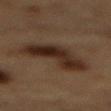Q: Was a biopsy performed?
A: imaged on a skin check; not biopsied
Q: Automated lesion metrics?
A: a footprint of about 20 mm², a shape eccentricity near 0.9, and a shape-asymmetry score of about 0.4 (0 = symmetric); an average lesion color of about L≈23 a*≈14 b*≈21 (CIELAB) and a normalized lesion–skin contrast near 11; a border-irregularity index near 4.5/10, a color-variation rating of about 5/10, and a peripheral color-asymmetry measure near 1.5; an automated nevus-likeness rating near 90 out of 100
Q: Where on the body is the lesion?
A: the back
Q: Illumination type?
A: cross-polarized
Q: How large is the lesion?
A: about 7.5 mm
Q: Patient demographics?
A: male, aged around 85 or older
Q: What is the imaging modality?
A: 15 mm crop, total-body photography